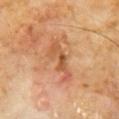<lesion>
<biopsy_status>not biopsied; imaged during a skin examination</biopsy_status>
<image>
  <source>total-body photography crop</source>
  <field_of_view_mm>15</field_of_view_mm>
</image>
<patient>
  <sex>male</sex>
  <age_approx>60</age_approx>
</patient>
<lesion_size>
  <long_diameter_mm_approx>6.0</long_diameter_mm_approx>
</lesion_size>
<lighting>cross-polarized</lighting>
<site>front of the torso</site>
<automated_metrics>
  <area_mm2_approx>9.5</area_mm2_approx>
  <vs_skin_darker_L>10.0</vs_skin_darker_L>
</automated_metrics>
</lesion>